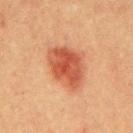Part of a total-body skin-imaging series; this lesion was reviewed on a skin check and was not flagged for biopsy.
An algorithmic analysis of the crop reported border irregularity of about 2 on a 0–10 scale, a color-variation rating of about 4.5/10, and a peripheral color-asymmetry measure near 1.5.
Approximately 6 mm at its widest.
Located on the mid back.
The subject is a male aged around 40.
Imaged with cross-polarized lighting.
A 15 mm close-up extracted from a 3D total-body photography capture.